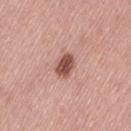Image and clinical context: This is a white-light tile. The lesion's longest dimension is about 3 mm. Cropped from a total-body skin-imaging series; the visible field is about 15 mm. A female patient aged 38–42. On the left thigh.